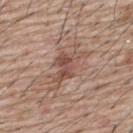Part of a total-body skin-imaging series; this lesion was reviewed on a skin check and was not flagged for biopsy. Located on the upper back. The lesion-visualizer software estimated an area of roughly 9.5 mm² and an outline eccentricity of about 0.85 (0 = round, 1 = elongated). The software also gave an average lesion color of about L≈51 a*≈20 b*≈25 (CIELAB). The software also gave a border-irregularity rating of about 6.5/10, a within-lesion color-variation index near 4.5/10, and radial color variation of about 1.5. The software also gave a classifier nevus-likeness of about 0/100 and a detector confidence of about 100 out of 100 that the crop contains a lesion. This image is a 15 mm lesion crop taken from a total-body photograph. The tile uses white-light illumination. A male subject, approximately 65 years of age. The lesion's longest dimension is about 6 mm.